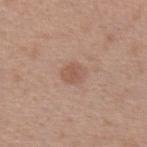<case>
  <biopsy_status>not biopsied; imaged during a skin examination</biopsy_status>
</case>